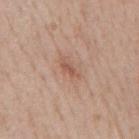The lesion was tiled from a total-body skin photograph and was not biopsied. The lesion is on the mid back. A 15 mm close-up extracted from a 3D total-body photography capture. Longest diameter approximately 2.5 mm. Automated image analysis of the tile measured a footprint of about 2.5 mm², a shape eccentricity near 0.9, and a symmetry-axis asymmetry near 0.35. The software also gave a detector confidence of about 100 out of 100 that the crop contains a lesion. A male subject, about 50 years old. Imaged with white-light lighting.